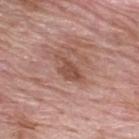Q: Is there a histopathology result?
A: catalogued during a skin exam; not biopsied
Q: How was this image acquired?
A: ~15 mm tile from a whole-body skin photo
Q: Where on the body is the lesion?
A: the upper back
Q: Patient demographics?
A: male, aged around 60
Q: What lighting was used for the tile?
A: white-light illumination
Q: What did automated image analysis measure?
A: border irregularity of about 4.5 on a 0–10 scale and internal color variation of about 1.5 on a 0–10 scale; a nevus-likeness score of about 0/100 and a lesion-detection confidence of about 100/100